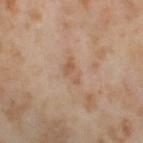notes: total-body-photography surveillance lesion; no biopsy
subject: female, roughly 55 years of age
tile lighting: cross-polarized
imaging modality: total-body-photography crop, ~15 mm field of view
image-analysis metrics: a detector confidence of about 100 out of 100 that the crop contains a lesion
site: the right thigh